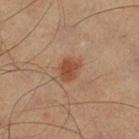Recorded during total-body skin imaging; not selected for excision or biopsy.
The lesion is on the right lower leg.
A 15 mm close-up tile from a total-body photography series done for melanoma screening.
A male patient aged around 60.
About 3 mm across.